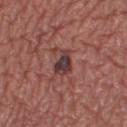Clinical impression:
Captured during whole-body skin photography for melanoma surveillance; the lesion was not biopsied.
Background:
About 3 mm across. On the right thigh. The subject is a female aged around 60. Cropped from a total-body skin-imaging series; the visible field is about 15 mm. The tile uses white-light illumination.